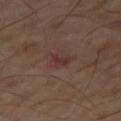No biopsy was performed on this lesion — it was imaged during a full skin examination and was not determined to be concerning.
Automated tile analysis of the lesion measured a classifier nevus-likeness of about 0/100 and a detector confidence of about 100 out of 100 that the crop contains a lesion.
Located on the right thigh.
Imaged with cross-polarized lighting.
A close-up tile cropped from a whole-body skin photograph, about 15 mm across.
Approximately 3 mm at its widest.